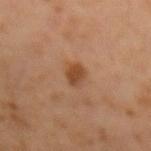Clinical impression: Recorded during total-body skin imaging; not selected for excision or biopsy. Acquisition and patient details: A male patient roughly 60 years of age. On the left forearm. A 15 mm close-up tile from a total-body photography series done for melanoma screening. The lesion-visualizer software estimated a lesion color around L≈42 a*≈21 b*≈33 in CIELAB. It also reported a within-lesion color-variation index near 3/10 and radial color variation of about 1. The software also gave a detector confidence of about 100 out of 100 that the crop contains a lesion. This is a cross-polarized tile.